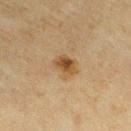Q: Was a biopsy performed?
A: imaged on a skin check; not biopsied
Q: Where on the body is the lesion?
A: the leg
Q: Automated lesion metrics?
A: a lesion–skin lightness drop of about 9 and a normalized lesion–skin contrast near 8; border irregularity of about 2 on a 0–10 scale, a color-variation rating of about 5/10, and peripheral color asymmetry of about 1.5; a classifier nevus-likeness of about 85/100 and a detector confidence of about 100 out of 100 that the crop contains a lesion
Q: Lesion size?
A: ≈3 mm
Q: How was the tile lit?
A: cross-polarized
Q: What is the imaging modality?
A: total-body-photography crop, ~15 mm field of view
Q: Who is the patient?
A: female, aged 53 to 57